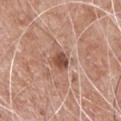A 15 mm close-up tile from a total-body photography series done for melanoma screening.
The lesion is located on the chest.
The subject is a male aged 63–67.